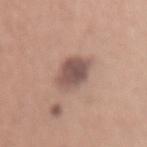This lesion was catalogued during total-body skin photography and was not selected for biopsy.
A male patient about 45 years old.
A 15 mm close-up extracted from a 3D total-body photography capture.
Captured under white-light illumination.
The lesion is located on the abdomen.
The lesion-visualizer software estimated an average lesion color of about L≈52 a*≈18 b*≈22 (CIELAB). And it measured a border-irregularity rating of about 1.5/10 and peripheral color asymmetry of about 1. And it measured a nevus-likeness score of about 30/100 and a lesion-detection confidence of about 100/100.
The lesion's longest dimension is about 3.5 mm.